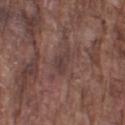{"biopsy_status": "not biopsied; imaged during a skin examination", "lesion_size": {"long_diameter_mm_approx": 2.5}, "site": "left upper arm", "patient": {"sex": "male", "age_approx": 75}, "image": {"source": "total-body photography crop", "field_of_view_mm": 15}}